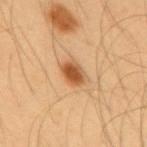Case summary:
• workup · total-body-photography surveillance lesion; no biopsy
• lighting · cross-polarized illumination
• lesion size · ~3 mm (longest diameter)
• imaging modality · 15 mm crop, total-body photography
• subject · male, aged around 55
• body site · the mid back